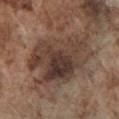The lesion was photographed on a routine skin check and not biopsied; there is no pathology result. On the chest. A male subject in their mid- to late 70s. A 15 mm crop from a total-body photograph taken for skin-cancer surveillance.